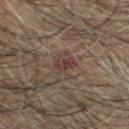Q: What is the lesion's diameter?
A: about 3.5 mm
Q: What is the imaging modality?
A: 15 mm crop, total-body photography
Q: What are the patient's age and sex?
A: male, approximately 70 years of age
Q: What is the anatomic site?
A: the head or neck